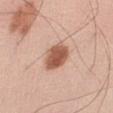Q: Was a biopsy performed?
A: total-body-photography surveillance lesion; no biopsy
Q: What is the lesion's diameter?
A: ≈3.5 mm
Q: What is the anatomic site?
A: the abdomen
Q: What is the imaging modality?
A: ~15 mm crop, total-body skin-cancer survey
Q: Patient demographics?
A: male, roughly 70 years of age